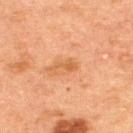Impression:
The lesion was photographed on a routine skin check and not biopsied; there is no pathology result.
Image and clinical context:
On the back. A 15 mm close-up extracted from a 3D total-body photography capture. The total-body-photography lesion software estimated a lesion area of about 2.5 mm². The analysis additionally found a lesion color around L≈47 a*≈21 b*≈34 in CIELAB and a lesion-to-skin contrast of about 6 (normalized; higher = more distinct). The lesion's longest dimension is about 2.5 mm. A male subject, aged 48–52.